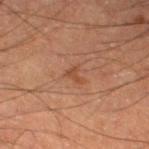  patient:
    sex: male
    age_approx: 60
  site: left thigh
  automated_metrics:
    area_mm2_approx: 2.0
    cielab_L: 43
    cielab_a: 22
    cielab_b: 31
    vs_skin_darker_L: 7.0
    vs_skin_contrast_norm: 6.0
    border_irregularity_0_10: 6.0
    peripheral_color_asymmetry: 0.0
    nevus_likeness_0_100: 0
    lesion_detection_confidence_0_100: 100
  lighting: cross-polarized
  lesion_size:
    long_diameter_mm_approx: 2.0
  image:
    source: total-body photography crop
    field_of_view_mm: 15